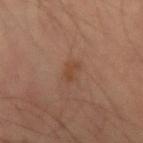This lesion was catalogued during total-body skin photography and was not selected for biopsy.
Automated tile analysis of the lesion measured a lesion color around L≈39 a*≈19 b*≈28 in CIELAB.
Measured at roughly 2.5 mm in maximum diameter.
Located on the right forearm.
This is a cross-polarized tile.
The subject is a male approximately 35 years of age.
Cropped from a whole-body photographic skin survey; the tile spans about 15 mm.